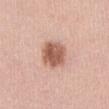notes — catalogued during a skin exam; not biopsied | size — about 3.5 mm | TBP lesion metrics — a mean CIELAB color near L≈58 a*≈23 b*≈29, about 15 CIELAB-L* units darker than the surrounding skin, and a normalized lesion–skin contrast near 9.5; a border-irregularity index near 1.5/10 and peripheral color asymmetry of about 1.5; a lesion-detection confidence of about 100/100 | anatomic site — the abdomen | patient — female, roughly 45 years of age | acquisition — ~15 mm crop, total-body skin-cancer survey | tile lighting — white-light illumination.